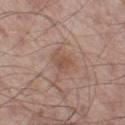  biopsy_status: not biopsied; imaged during a skin examination
  site: left thigh
  lighting: white-light
  image:
    source: total-body photography crop
    field_of_view_mm: 15
  patient:
    sex: male
    age_approx: 55
  lesion_size:
    long_diameter_mm_approx: 3.0